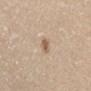acquisition = 15 mm crop, total-body photography; anatomic site = the back; patient = female, about 60 years old; size = about 3 mm.